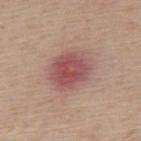Notes:
- notes · total-body-photography surveillance lesion; no biopsy
- subject · male, about 55 years old
- body site · the upper back
- tile lighting · white-light
- TBP lesion metrics · an outline eccentricity of about 0.6 (0 = round, 1 = elongated) and two-axis asymmetry of about 0.15; a classifier nevus-likeness of about 5/100 and a detector confidence of about 100 out of 100 that the crop contains a lesion
- lesion diameter · ≈5 mm
- image source · ~15 mm crop, total-body skin-cancer survey